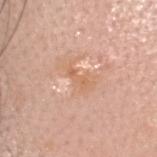Assessment: Part of a total-body skin-imaging series; this lesion was reviewed on a skin check and was not flagged for biopsy. Acquisition and patient details: On the head or neck. The tile uses white-light illumination. A male subject, aged 33–37. The total-body-photography lesion software estimated a mean CIELAB color near L≈64 a*≈22 b*≈34, a lesion–skin lightness drop of about 6, and a normalized lesion–skin contrast near 5. The analysis additionally found a border-irregularity index near 5/10, a color-variation rating of about 0/10, and radial color variation of about 0. The recorded lesion diameter is about 2.5 mm. A 15 mm close-up extracted from a 3D total-body photography capture.